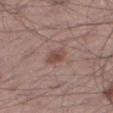biopsy status = imaged on a skin check; not biopsied | site = the right thigh | lesion size = ≈3 mm | image source = total-body-photography crop, ~15 mm field of view | image-analysis metrics = border irregularity of about 2 on a 0–10 scale, a color-variation rating of about 3/10, and a peripheral color-asymmetry measure near 1 | tile lighting = white-light illumination | subject = male, aged 53 to 57.